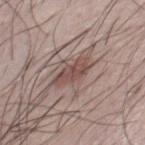Notes:
• workup: catalogued during a skin exam; not biopsied
• lesion diameter: about 4.5 mm
• location: the chest
• image source: total-body-photography crop, ~15 mm field of view
• patient: male, aged 48 to 52
• tile lighting: white-light